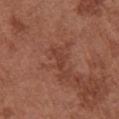The lesion-visualizer software estimated an outline eccentricity of about 0.95 (0 = round, 1 = elongated). The analysis additionally found a detector confidence of about 90 out of 100 that the crop contains a lesion.
A lesion tile, about 15 mm wide, cut from a 3D total-body photograph.
The tile uses white-light illumination.
The lesion is on the left arm.
A female patient, aged 63–67.